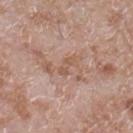biopsy status = catalogued during a skin exam; not biopsied | diameter = about 2.5 mm | site = the right lower leg | automated metrics = a within-lesion color-variation index near 0/10 and radial color variation of about 0; a lesion-detection confidence of about 90/100 | subject = male, approximately 60 years of age | imaging modality = ~15 mm tile from a whole-body skin photo | tile lighting = white-light.